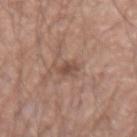Impression:
Recorded during total-body skin imaging; not selected for excision or biopsy.
Image and clinical context:
About 2.5 mm across. The subject is a male roughly 55 years of age. The total-body-photography lesion software estimated a footprint of about 3 mm², an eccentricity of roughly 0.75, and a symmetry-axis asymmetry near 0.25. The analysis additionally found an average lesion color of about L≈48 a*≈19 b*≈25 (CIELAB), roughly 9 lightness units darker than nearby skin, and a lesion-to-skin contrast of about 7 (normalized; higher = more distinct). And it measured lesion-presence confidence of about 100/100. A 15 mm crop from a total-body photograph taken for skin-cancer surveillance.